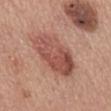Imaged during a routine full-body skin examination; the lesion was not biopsied and no histopathology is available. The lesion is on the mid back. A region of skin cropped from a whole-body photographic capture, roughly 15 mm wide. The subject is a male aged around 55.